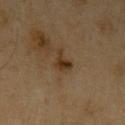Impression:
The lesion was tiled from a total-body skin photograph and was not biopsied.
Context:
The lesion is located on the arm. The recorded lesion diameter is about 2.5 mm. This image is a 15 mm lesion crop taken from a total-body photograph. A male patient, in their 70s.